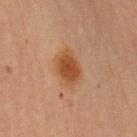notes — total-body-photography surveillance lesion; no biopsy
patient — female, in their 70s
illumination — cross-polarized illumination
location — the left upper arm
image — ~15 mm crop, total-body skin-cancer survey
diameter — about 4 mm